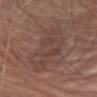biopsy status = total-body-photography surveillance lesion; no biopsy | subject = male, aged approximately 75 | image = ~15 mm crop, total-body skin-cancer survey | location = the abdomen | size = ≈10 mm | tile lighting = white-light illumination.